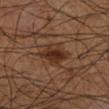biopsy status: total-body-photography surveillance lesion; no biopsy | patient: male, roughly 60 years of age | image source: 15 mm crop, total-body photography | anatomic site: the leg | size: about 3 mm | illumination: cross-polarized | TBP lesion metrics: an eccentricity of roughly 0.3 and a shape-asymmetry score of about 0.15 (0 = symmetric); a mean CIELAB color near L≈30 a*≈19 b*≈27, about 9 CIELAB-L* units darker than the surrounding skin, and a normalized lesion–skin contrast near 8.5; a classifier nevus-likeness of about 90/100 and a detector confidence of about 100 out of 100 that the crop contains a lesion.